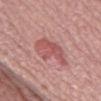Recorded during total-body skin imaging; not selected for excision or biopsy. About 4.5 mm across. A close-up tile cropped from a whole-body skin photograph, about 15 mm across. Captured under white-light illumination. The lesion-visualizer software estimated an eccentricity of roughly 0.65 and two-axis asymmetry of about 0.3. It also reported a lesion color around L≈55 a*≈28 b*≈23 in CIELAB and a normalized border contrast of about 6.5. The analysis additionally found a nevus-likeness score of about 40/100 and a lesion-detection confidence of about 100/100. The lesion is located on the leg. A female subject, approximately 45 years of age.